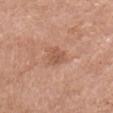  image:
    source: total-body photography crop
    field_of_view_mm: 15
  site: head or neck
  patient:
    sex: female
    age_approx: 70